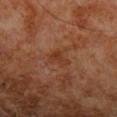workup: total-body-photography surveillance lesion; no biopsy | diameter: about 3 mm | acquisition: ~15 mm tile from a whole-body skin photo | patient: male, aged 68–72 | anatomic site: the left lower leg | automated metrics: an area of roughly 4.5 mm², an eccentricity of roughly 0.65, and two-axis asymmetry of about 0.5; an automated nevus-likeness rating near 0 out of 100 and lesion-presence confidence of about 100/100.